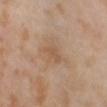Located on the lower back. A lesion tile, about 15 mm wide, cut from a 3D total-body photograph. A female patient, approximately 55 years of age. The tile uses cross-polarized illumination.